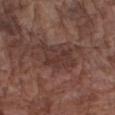Imaged during a routine full-body skin examination; the lesion was not biopsied and no histopathology is available. The total-body-photography lesion software estimated a border-irregularity rating of about 4.5/10, a within-lesion color-variation index near 3/10, and radial color variation of about 1. On the arm. Imaged with white-light lighting. A male patient aged 73–77. A 15 mm close-up extracted from a 3D total-body photography capture.